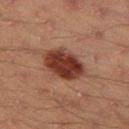The lesion was tiled from a total-body skin photograph and was not biopsied. A region of skin cropped from a whole-body photographic capture, roughly 15 mm wide. The lesion is located on the left thigh. The subject is a male in their mid-40s. The tile uses cross-polarized illumination.